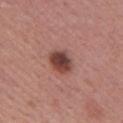This lesion was catalogued during total-body skin photography and was not selected for biopsy. The total-body-photography lesion software estimated an eccentricity of roughly 0.45 and a shape-asymmetry score of about 0.15 (0 = symmetric). The software also gave a border-irregularity rating of about 1.5/10, a within-lesion color-variation index near 5/10, and a peripheral color-asymmetry measure near 1.5. The recorded lesion diameter is about 3 mm. This is a white-light tile. A roughly 15 mm field-of-view crop from a total-body skin photograph. The subject is a female aged 48 to 52. From the arm.